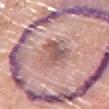Q: Was this lesion biopsied?
A: total-body-photography surveillance lesion; no biopsy
Q: Who is the patient?
A: male, in their 80s
Q: What is the imaging modality?
A: ~15 mm tile from a whole-body skin photo
Q: What did automated image analysis measure?
A: an area of roughly 13 mm², an outline eccentricity of about 0.65 (0 = round, 1 = elongated), and a symmetry-axis asymmetry near 0.25; border irregularity of about 3.5 on a 0–10 scale, internal color variation of about 7.5 on a 0–10 scale, and radial color variation of about 2.5; a classifier nevus-likeness of about 20/100
Q: Lesion location?
A: the chest
Q: What lighting was used for the tile?
A: white-light